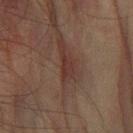workup: imaged on a skin check; not biopsied
subject: male, roughly 75 years of age
site: the left forearm
image source: 15 mm crop, total-body photography